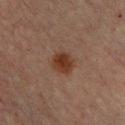Imaged during a routine full-body skin examination; the lesion was not biopsied and no histopathology is available.
The subject is a male aged around 60.
This is a cross-polarized tile.
A 15 mm close-up extracted from a 3D total-body photography capture.
Located on the chest.